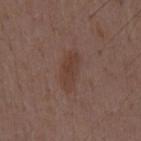Assessment:
The lesion was photographed on a routine skin check and not biopsied; there is no pathology result.
Clinical summary:
From the mid back. The recorded lesion diameter is about 4.5 mm. The patient is a male aged approximately 50. A 15 mm crop from a total-body photograph taken for skin-cancer surveillance. The total-body-photography lesion software estimated a border-irregularity rating of about 3/10, internal color variation of about 2.5 on a 0–10 scale, and a peripheral color-asymmetry measure near 1. This is a white-light tile.